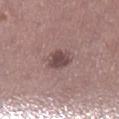Assessment: Recorded during total-body skin imaging; not selected for excision or biopsy.